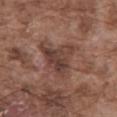Captured during whole-body skin photography for melanoma surveillance; the lesion was not biopsied.
A male patient, in their mid-70s.
The tile uses white-light illumination.
Cropped from a whole-body photographic skin survey; the tile spans about 15 mm.
The lesion is on the abdomen.
Approximately 5.5 mm at its widest.
The total-body-photography lesion software estimated a mean CIELAB color near L≈40 a*≈19 b*≈23 and a lesion-to-skin contrast of about 8 (normalized; higher = more distinct). And it measured a lesion-detection confidence of about 85/100.